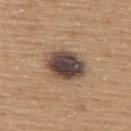<case>
<biopsy_status>not biopsied; imaged during a skin examination</biopsy_status>
<patient>
  <sex>male</sex>
  <age_approx>65</age_approx>
</patient>
<automated_metrics>
  <area_mm2_approx>14.0</area_mm2_approx>
  <shape_asymmetry>0.15</shape_asymmetry>
</automated_metrics>
<lighting>white-light</lighting>
<image>
  <source>total-body photography crop</source>
  <field_of_view_mm>15</field_of_view_mm>
</image>
<site>upper back</site>
<lesion_size>
  <long_diameter_mm_approx>5.0</long_diameter_mm_approx>
</lesion_size>
</case>